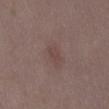biopsy_status: not biopsied; imaged during a skin examination
automated_metrics:
  area_mm2_approx: 3.0
  eccentricity: 0.8
  shape_asymmetry: 0.3
  nevus_likeness_0_100: 0
  lesion_detection_confidence_0_100: 100
image:
  source: total-body photography crop
  field_of_view_mm: 15
patient:
  sex: female
  age_approx: 35
lighting: white-light
lesion_size:
  long_diameter_mm_approx: 2.5
site: abdomen